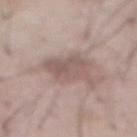workup: no biopsy performed (imaged during a skin exam); illumination: white-light illumination; lesion size: ≈4.5 mm; patient: male, roughly 55 years of age; anatomic site: the abdomen; imaging modality: total-body-photography crop, ~15 mm field of view.